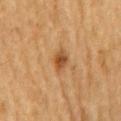This lesion was catalogued during total-body skin photography and was not selected for biopsy.
The patient is a male aged 83 or older.
The tile uses cross-polarized illumination.
Cropped from a whole-body photographic skin survey; the tile spans about 15 mm.
Located on the mid back.